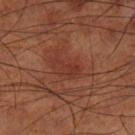notes=catalogued during a skin exam; not biopsied | patient=male, aged 68 to 72 | acquisition=total-body-photography crop, ~15 mm field of view | location=the left lower leg.